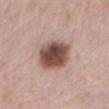biopsy status: no biopsy performed (imaged during a skin exam) | TBP lesion metrics: an area of roughly 15 mm², an outline eccentricity of about 0.65 (0 = round, 1 = elongated), and two-axis asymmetry of about 0.15; border irregularity of about 1.5 on a 0–10 scale, a within-lesion color-variation index near 6.5/10, and peripheral color asymmetry of about 1.5; lesion-presence confidence of about 100/100 | site: the mid back | patient: female, aged approximately 65 | acquisition: 15 mm crop, total-body photography | tile lighting: white-light illumination | lesion diameter: ≈5 mm.